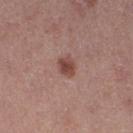Captured during whole-body skin photography for melanoma surveillance; the lesion was not biopsied.
From the right thigh.
The tile uses white-light illumination.
An algorithmic analysis of the crop reported an area of roughly 4 mm² and a shape eccentricity near 0.55. It also reported a mean CIELAB color near L≈46 a*≈23 b*≈24, a lesion–skin lightness drop of about 11, and a lesion-to-skin contrast of about 8.5 (normalized; higher = more distinct).
The patient is a female aged approximately 40.
A 15 mm crop from a total-body photograph taken for skin-cancer surveillance.